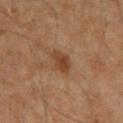Assessment:
Captured during whole-body skin photography for melanoma surveillance; the lesion was not biopsied.
Clinical summary:
On the front of the torso. Cropped from a total-body skin-imaging series; the visible field is about 15 mm. Captured under cross-polarized illumination. The subject is a male in their 60s.